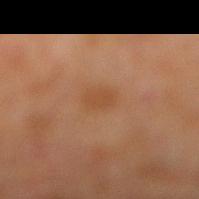lesion diameter = ≈2.5 mm
lighting = cross-polarized
patient = male, aged around 60
image source = ~15 mm crop, total-body skin-cancer survey
automated lesion analysis = a shape eccentricity near 0.55 and two-axis asymmetry of about 0.2
anatomic site = the right lower leg